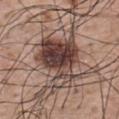Captured during whole-body skin photography for melanoma surveillance; the lesion was not biopsied. The lesion is located on the upper back. The lesion's longest dimension is about 8 mm. A male patient, aged approximately 65. A 15 mm close-up extracted from a 3D total-body photography capture. Imaged with white-light lighting.